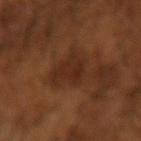Imaged with cross-polarized lighting. Approximately 4 mm at its widest. On the right forearm. Cropped from a whole-body photographic skin survey; the tile spans about 15 mm. The patient is a male aged approximately 65.